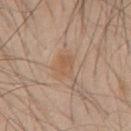- biopsy status: catalogued during a skin exam; not biopsied
- location: the chest
- TBP lesion metrics: about 6 CIELAB-L* units darker than the surrounding skin and a normalized border contrast of about 5.5; border irregularity of about 2.5 on a 0–10 scale, a color-variation rating of about 2.5/10, and a peripheral color-asymmetry measure near 1; a classifier nevus-likeness of about 10/100 and a lesion-detection confidence of about 100/100
- patient: male, aged 43–47
- size: ≈2.5 mm
- image source: ~15 mm tile from a whole-body skin photo
- illumination: white-light illumination